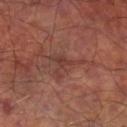follow-up: no biopsy performed (imaged during a skin exam)